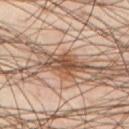follow-up: no biopsy performed (imaged during a skin exam)
image: 15 mm crop, total-body photography
patient: male, aged approximately 45
anatomic site: the front of the torso
lesion size: about 5.5 mm
tile lighting: cross-polarized
TBP lesion metrics: a mean CIELAB color near L≈49 a*≈19 b*≈30, about 15 CIELAB-L* units darker than the surrounding skin, and a normalized border contrast of about 10.5; a border-irregularity rating of about 5/10, internal color variation of about 5 on a 0–10 scale, and peripheral color asymmetry of about 2